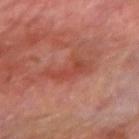| key | value |
|---|---|
| size | ≈5 mm |
| patient | male, roughly 70 years of age |
| lighting | cross-polarized |
| site | the right forearm |
| acquisition | ~15 mm tile from a whole-body skin photo |